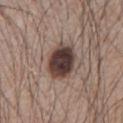| feature | finding |
|---|---|
| follow-up | total-body-photography surveillance lesion; no biopsy |
| acquisition | ~15 mm crop, total-body skin-cancer survey |
| subject | male, in their mid- to late 60s |
| location | the chest |